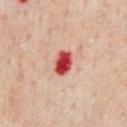  biopsy_status: not biopsied; imaged during a skin examination
  image:
    source: total-body photography crop
    field_of_view_mm: 15
  patient:
    sex: male
    age_approx: 65
  site: chest